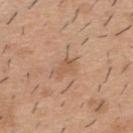Captured during whole-body skin photography for melanoma surveillance; the lesion was not biopsied.
Measured at roughly 2.5 mm in maximum diameter.
An algorithmic analysis of the crop reported a lesion area of about 3.5 mm², a shape eccentricity near 0.85, and two-axis asymmetry of about 0.35. It also reported an average lesion color of about L≈57 a*≈20 b*≈33 (CIELAB), about 7 CIELAB-L* units darker than the surrounding skin, and a normalized lesion–skin contrast near 5. It also reported a border-irregularity index near 3.5/10, a within-lesion color-variation index near 1.5/10, and radial color variation of about 0.5. The analysis additionally found an automated nevus-likeness rating near 0 out of 100 and lesion-presence confidence of about 100/100.
A roughly 15 mm field-of-view crop from a total-body skin photograph.
Captured under white-light illumination.
A male patient aged approximately 40.
Located on the mid back.